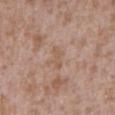Impression: Recorded during total-body skin imaging; not selected for excision or biopsy. Clinical summary: On the mid back. This image is a 15 mm lesion crop taken from a total-body photograph. A male patient, about 45 years old.